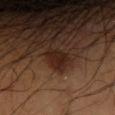Q: Was this lesion biopsied?
A: total-body-photography surveillance lesion; no biopsy
Q: How large is the lesion?
A: about 3 mm
Q: What is the anatomic site?
A: the arm
Q: What lighting was used for the tile?
A: cross-polarized illumination
Q: Who is the patient?
A: male, about 60 years old
Q: How was this image acquired?
A: total-body-photography crop, ~15 mm field of view